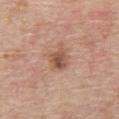Q: Is there a histopathology result?
A: total-body-photography surveillance lesion; no biopsy
Q: Who is the patient?
A: male, about 70 years old
Q: What did automated image analysis measure?
A: a mean CIELAB color near L≈53 a*≈20 b*≈30 and about 11 CIELAB-L* units darker than the surrounding skin
Q: What is the anatomic site?
A: the abdomen
Q: How was the tile lit?
A: white-light
Q: How was this image acquired?
A: total-body-photography crop, ~15 mm field of view